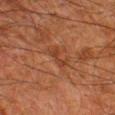| feature | finding |
|---|---|
| workup | total-body-photography surveillance lesion; no biopsy |
| patient | male, aged around 80 |
| lesion diameter | ~3 mm (longest diameter) |
| acquisition | ~15 mm crop, total-body skin-cancer survey |
| site | the left thigh |
| image-analysis metrics | a footprint of about 2.5 mm², an eccentricity of roughly 0.9, and two-axis asymmetry of about 0.55; a mean CIELAB color near L≈31 a*≈21 b*≈28; a border-irregularity index near 6.5/10, a color-variation rating of about 0/10, and a peripheral color-asymmetry measure near 0 |